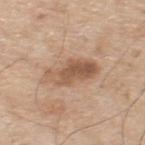notes — total-body-photography surveillance lesion; no biopsy
lesion size — ~6 mm (longest diameter)
body site — the upper back
subject — male, approximately 70 years of age
imaging modality — ~15 mm crop, total-body skin-cancer survey